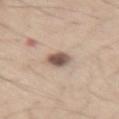biopsy status: total-body-photography surveillance lesion; no biopsy.